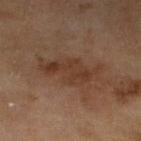Imaged during a routine full-body skin examination; the lesion was not biopsied and no histopathology is available.
A 15 mm close-up extracted from a 3D total-body photography capture.
Measured at roughly 7 mm in maximum diameter.
From the left lower leg.
A female patient, in their 60s.